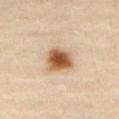The lesion was photographed on a routine skin check and not biopsied; there is no pathology result.
The recorded lesion diameter is about 3.5 mm.
A female subject roughly 65 years of age.
From the abdomen.
Imaged with cross-polarized lighting.
Cropped from a whole-body photographic skin survey; the tile spans about 15 mm.